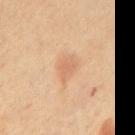| field | value |
|---|---|
| automated lesion analysis | an area of roughly 4 mm², an outline eccentricity of about 0.75 (0 = round, 1 = elongated), and two-axis asymmetry of about 0.25; an average lesion color of about L≈63 a*≈22 b*≈35 (CIELAB); a border-irregularity index near 2.5/10, a within-lesion color-variation index near 1/10, and a peripheral color-asymmetry measure near 0.5 |
| subject | female, about 65 years old |
| image | 15 mm crop, total-body photography |
| location | the front of the torso |